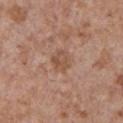The lesion was photographed on a routine skin check and not biopsied; there is no pathology result. Located on the chest. A male patient, roughly 65 years of age. A lesion tile, about 15 mm wide, cut from a 3D total-body photograph. The tile uses white-light illumination. The lesion-visualizer software estimated a mean CIELAB color near L≈51 a*≈20 b*≈30, roughly 8 lightness units darker than nearby skin, and a lesion-to-skin contrast of about 6 (normalized; higher = more distinct). It also reported border irregularity of about 2.5 on a 0–10 scale and a peripheral color-asymmetry measure near 1. Approximately 3 mm at its widest.